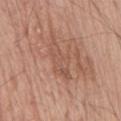{"biopsy_status": "not biopsied; imaged during a skin examination", "site": "abdomen", "automated_metrics": {"area_mm2_approx": 9.0, "shape_asymmetry": 0.35, "cielab_L": 54, "cielab_a": 21, "cielab_b": 28, "vs_skin_darker_L": 7.0, "vs_skin_contrast_norm": 4.5}, "lighting": "white-light", "patient": {"sex": "male", "age_approx": 70}, "image": {"source": "total-body photography crop", "field_of_view_mm": 15}}